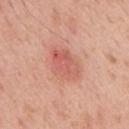Case summary:
• notes — catalogued during a skin exam; not biopsied
• subject — male, aged 53 to 57
• size — about 5 mm
• lighting — white-light illumination
• anatomic site — the mid back
• image — ~15 mm tile from a whole-body skin photo